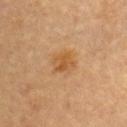Assessment: Recorded during total-body skin imaging; not selected for excision or biopsy. Context: Approximately 3 mm at its widest. The lesion-visualizer software estimated a footprint of about 6 mm², a shape eccentricity near 0.55, and a shape-asymmetry score of about 0.2 (0 = symmetric). The patient is a female aged around 60. A close-up tile cropped from a whole-body skin photograph, about 15 mm across. From the front of the torso.